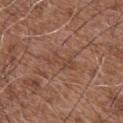Findings:
- workup: total-body-photography surveillance lesion; no biopsy
- patient: male, in their mid- to late 70s
- automated metrics: a lesion area of about 5.5 mm², an outline eccentricity of about 0.9 (0 = round, 1 = elongated), and a shape-asymmetry score of about 0.4 (0 = symmetric)
- illumination: white-light illumination
- anatomic site: the chest
- diameter: ~4 mm (longest diameter)
- imaging modality: total-body-photography crop, ~15 mm field of view